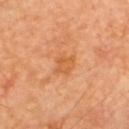Part of a total-body skin-imaging series; this lesion was reviewed on a skin check and was not flagged for biopsy.
The lesion is located on the upper back.
Cropped from a whole-body photographic skin survey; the tile spans about 15 mm.
Imaged with cross-polarized lighting.
Longest diameter approximately 3 mm.
A male patient aged 58 to 62.
Automated tile analysis of the lesion measured a border-irregularity index near 2/10, a within-lesion color-variation index near 3/10, and a peripheral color-asymmetry measure near 1.